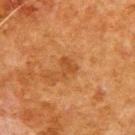The lesion was tiled from a total-body skin photograph and was not biopsied.
Measured at roughly 2.5 mm in maximum diameter.
From the upper back.
The subject is a male aged approximately 80.
Captured under cross-polarized illumination.
A 15 mm crop from a total-body photograph taken for skin-cancer surveillance.